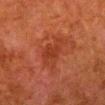A region of skin cropped from a whole-body photographic capture, roughly 15 mm wide.
On the left lower leg.
The lesion's longest dimension is about 4.5 mm.
A male subject, about 80 years old.
Imaged with cross-polarized lighting.